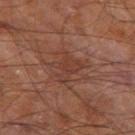Q: Was a biopsy performed?
A: imaged on a skin check; not biopsied
Q: Who is the patient?
A: male, about 60 years old
Q: How was this image acquired?
A: 15 mm crop, total-body photography
Q: What is the anatomic site?
A: the leg
Q: How was the tile lit?
A: cross-polarized
Q: Automated lesion metrics?
A: a lesion area of about 7 mm², an outline eccentricity of about 0.6 (0 = round, 1 = elongated), and a symmetry-axis asymmetry near 0.5; an average lesion color of about L≈37 a*≈21 b*≈26 (CIELAB) and about 6 CIELAB-L* units darker than the surrounding skin; an automated nevus-likeness rating near 0 out of 100 and lesion-presence confidence of about 95/100